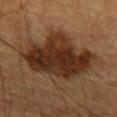Q: Was this lesion biopsied?
A: catalogued during a skin exam; not biopsied
Q: What are the patient's age and sex?
A: male, aged around 85 or older
Q: Where on the body is the lesion?
A: the right upper arm
Q: Automated lesion metrics?
A: a footprint of about 36 mm² and a shape-asymmetry score of about 0.25 (0 = symmetric); roughly 12 lightness units darker than nearby skin
Q: What is the imaging modality?
A: total-body-photography crop, ~15 mm field of view
Q: Illumination type?
A: cross-polarized illumination